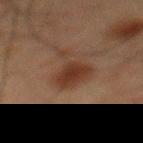biopsy status: total-body-photography surveillance lesion; no biopsy
body site: the mid back
patient: male, roughly 60 years of age
acquisition: 15 mm crop, total-body photography